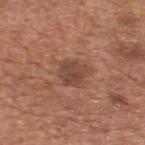biopsy status: catalogued during a skin exam; not biopsied | location: the upper back | size: ~3.5 mm (longest diameter) | subject: male, approximately 65 years of age | image source: 15 mm crop, total-body photography.